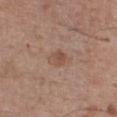biopsy status=imaged on a skin check; not biopsied | body site=the front of the torso | acquisition=total-body-photography crop, ~15 mm field of view | lighting=white-light illumination | subject=male, approximately 45 years of age.